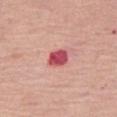Acquisition and patient details:
The lesion is on the right thigh. A female patient aged 63–67. A region of skin cropped from a whole-body photographic capture, roughly 15 mm wide.